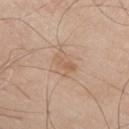<tbp_lesion>
  <biopsy_status>not biopsied; imaged during a skin examination</biopsy_status>
  <automated_metrics>
    <area_mm2_approx>6.0</area_mm2_approx>
    <eccentricity>0.75</eccentricity>
    <shape_asymmetry>0.25</shape_asymmetry>
    <cielab_L>60</cielab_L>
    <cielab_a>17</cielab_a>
    <cielab_b>31</cielab_b>
    <vs_skin_darker_L>7.0</vs_skin_darker_L>
    <vs_skin_contrast_norm>5.0</vs_skin_contrast_norm>
    <border_irregularity_0_10>2.5</border_irregularity_0_10>
    <color_variation_0_10>3.5</color_variation_0_10>
    <lesion_detection_confidence_0_100>100</lesion_detection_confidence_0_100>
  </automated_metrics>
  <lesion_size>
    <long_diameter_mm_approx>3.5</long_diameter_mm_approx>
  </lesion_size>
  <site>chest</site>
  <lighting>white-light</lighting>
  <image>
    <source>total-body photography crop</source>
    <field_of_view_mm>15</field_of_view_mm>
  </image>
  <patient>
    <sex>male</sex>
    <age_approx>80</age_approx>
  </patient>
</tbp_lesion>